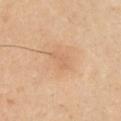workup=catalogued during a skin exam; not biopsied | lesion diameter=~3 mm (longest diameter) | site=the right upper arm | tile lighting=cross-polarized illumination | acquisition=~15 mm crop, total-body skin-cancer survey | patient=male, aged 43–47.